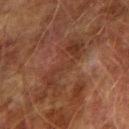Q: Was a biopsy performed?
A: no biopsy performed (imaged during a skin exam)
Q: What is the anatomic site?
A: the right upper arm
Q: How was this image acquired?
A: 15 mm crop, total-body photography
Q: What are the patient's age and sex?
A: male, roughly 75 years of age